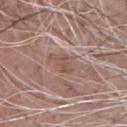notes: catalogued during a skin exam; not biopsied | imaging modality: ~15 mm crop, total-body skin-cancer survey | size: ~3.5 mm (longest diameter) | subject: male, about 70 years old | automated metrics: a lesion color around L≈53 a*≈18 b*≈25 in CIELAB, about 7 CIELAB-L* units darker than the surrounding skin, and a lesion-to-skin contrast of about 5.5 (normalized; higher = more distinct); border irregularity of about 2.5 on a 0–10 scale, internal color variation of about 3 on a 0–10 scale, and a peripheral color-asymmetry measure near 1 | illumination: white-light illumination | anatomic site: the front of the torso.